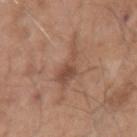Notes:
– biopsy status: imaged on a skin check; not biopsied
– lesion diameter: ≈5 mm
– location: the arm
– tile lighting: white-light
– acquisition: ~15 mm crop, total-body skin-cancer survey
– subject: male, aged around 50